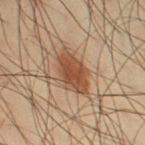No biopsy was performed on this lesion — it was imaged during a full skin examination and was not determined to be concerning. A 15 mm crop from a total-body photograph taken for skin-cancer surveillance. The subject is a male roughly 35 years of age. The lesion is on the right thigh.